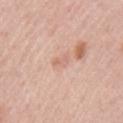Captured during whole-body skin photography for melanoma surveillance; the lesion was not biopsied. Cropped from a total-body skin-imaging series; the visible field is about 15 mm. On the arm. This is a white-light tile. Longest diameter approximately 2.5 mm. The subject is a male aged approximately 55.